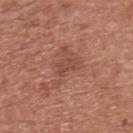This lesion was catalogued during total-body skin photography and was not selected for biopsy.
Longest diameter approximately 2.5 mm.
A lesion tile, about 15 mm wide, cut from a 3D total-body photograph.
The lesion is on the chest.
The subject is a male in their mid- to late 40s.